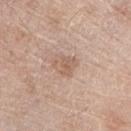Findings:
• notes — imaged on a skin check; not biopsied
• patient — male, approximately 80 years of age
• automated metrics — border irregularity of about 2.5 on a 0–10 scale and a color-variation rating of about 2.5/10; an automated nevus-likeness rating near 0 out of 100 and a detector confidence of about 100 out of 100 that the crop contains a lesion
• tile lighting — white-light
• imaging modality — total-body-photography crop, ~15 mm field of view
• location — the right upper arm